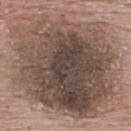Case summary:
• follow-up · total-body-photography surveillance lesion; no biopsy
• subject · male, aged 53 to 57
• acquisition · ~15 mm tile from a whole-body skin photo
• TBP lesion metrics · an area of roughly 110 mm², an eccentricity of roughly 0.65, and a shape-asymmetry score of about 0.15 (0 = symmetric); an average lesion color of about L≈47 a*≈14 b*≈22 (CIELAB) and a lesion-to-skin contrast of about 10 (normalized; higher = more distinct)
• tile lighting · white-light
• location · the upper back